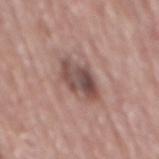* anatomic site · the mid back
* acquisition · total-body-photography crop, ~15 mm field of view
* subject · male, approximately 70 years of age